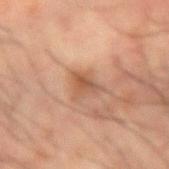Assessment:
No biopsy was performed on this lesion — it was imaged during a full skin examination and was not determined to be concerning.
Context:
A male patient aged 58–62. A 15 mm close-up tile from a total-body photography series done for melanoma screening. From the right forearm.